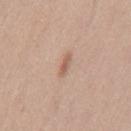The recorded lesion diameter is about 2.5 mm. This is a white-light tile. A male patient, in their mid- to late 40s. Located on the mid back. A lesion tile, about 15 mm wide, cut from a 3D total-body photograph.